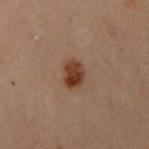This lesion was catalogued during total-body skin photography and was not selected for biopsy. An algorithmic analysis of the crop reported a lesion area of about 6.5 mm², an outline eccentricity of about 0.65 (0 = round, 1 = elongated), and two-axis asymmetry of about 0.2. And it measured an average lesion color of about L≈30 a*≈17 b*≈24 (CIELAB) and a lesion-to-skin contrast of about 10.5 (normalized; higher = more distinct). The tile uses cross-polarized illumination. A female subject, roughly 30 years of age. On the left arm. A 15 mm crop from a total-body photograph taken for skin-cancer surveillance. Approximately 3.5 mm at its widest.